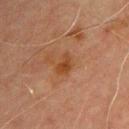Impression: Part of a total-body skin-imaging series; this lesion was reviewed on a skin check and was not flagged for biopsy. Background: The patient is a male aged approximately 70. A 15 mm crop from a total-body photograph taken for skin-cancer surveillance. Located on the front of the torso. Captured under cross-polarized illumination. Longest diameter approximately 2.5 mm.